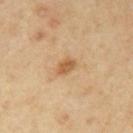Impression: The lesion was tiled from a total-body skin photograph and was not biopsied. Image and clinical context: A male subject aged approximately 55. On the right upper arm. Captured under cross-polarized illumination. About 2.5 mm across. The total-body-photography lesion software estimated a footprint of about 3.5 mm², an outline eccentricity of about 0.8 (0 = round, 1 = elongated), and two-axis asymmetry of about 0.2. The analysis additionally found an average lesion color of about L≈59 a*≈20 b*≈39 (CIELAB), a lesion–skin lightness drop of about 11, and a normalized border contrast of about 7.5. The analysis additionally found a border-irregularity rating of about 2/10, a within-lesion color-variation index near 2/10, and radial color variation of about 0.5. A region of skin cropped from a whole-body photographic capture, roughly 15 mm wide.